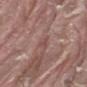Impression:
Captured during whole-body skin photography for melanoma surveillance; the lesion was not biopsied.
Context:
A male patient, in their 40s. On the lower back. A roughly 15 mm field-of-view crop from a total-body skin photograph. Imaged with white-light lighting.